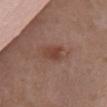| field | value |
|---|---|
| biopsy status | imaged on a skin check; not biopsied |
| diameter | ≈2.5 mm |
| acquisition | ~15 mm crop, total-body skin-cancer survey |
| subject | female, about 80 years old |
| site | the chest |